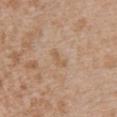No biopsy was performed on this lesion — it was imaged during a full skin examination and was not determined to be concerning.
The lesion's longest dimension is about 2.5 mm.
The lesion is on the chest.
A female subject roughly 30 years of age.
A roughly 15 mm field-of-view crop from a total-body skin photograph.
Captured under white-light illumination.